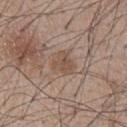Automated tile analysis of the lesion measured a footprint of about 5 mm² and two-axis asymmetry of about 0.45. And it measured an average lesion color of about L≈50 a*≈16 b*≈26 (CIELAB) and a normalized border contrast of about 6. The analysis additionally found border irregularity of about 5 on a 0–10 scale, a color-variation rating of about 2/10, and peripheral color asymmetry of about 1. A 15 mm crop from a total-body photograph taken for skin-cancer surveillance. A male patient, aged around 55. The lesion is located on the chest. Measured at roughly 3 mm in maximum diameter.